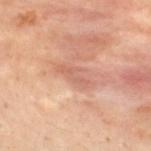Clinical impression: The lesion was tiled from a total-body skin photograph and was not biopsied. Clinical summary: The lesion is located on the mid back. A male patient, about 30 years old. A region of skin cropped from a whole-body photographic capture, roughly 15 mm wide. Imaged with cross-polarized lighting. The total-body-photography lesion software estimated roughly 6 lightness units darker than nearby skin and a normalized border contrast of about 4.5.